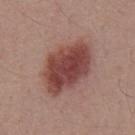Impression: Imaged during a routine full-body skin examination; the lesion was not biopsied and no histopathology is available. Acquisition and patient details: On the mid back. Approximately 7 mm at its widest. A male patient, roughly 55 years of age. Cropped from a total-body skin-imaging series; the visible field is about 15 mm. This is a white-light tile.